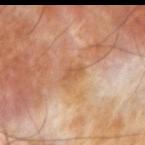Captured during whole-body skin photography for melanoma surveillance; the lesion was not biopsied. A close-up tile cropped from a whole-body skin photograph, about 15 mm across. On the left forearm. A male subject approximately 70 years of age.